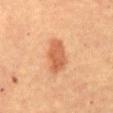Captured during whole-body skin photography for melanoma surveillance; the lesion was not biopsied. A close-up tile cropped from a whole-body skin photograph, about 15 mm across. The patient is a female roughly 60 years of age. This is a cross-polarized tile. From the abdomen.